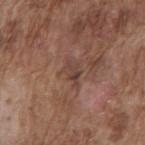The lesion was photographed on a routine skin check and not biopsied; there is no pathology result. A male subject, roughly 75 years of age. A 15 mm close-up tile from a total-body photography series done for melanoma screening. The tile uses white-light illumination. Located on the back. Measured at roughly 3 mm in maximum diameter.